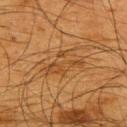follow-up: total-body-photography surveillance lesion; no biopsy
site: the upper back
imaging modality: ~15 mm tile from a whole-body skin photo
tile lighting: cross-polarized illumination
subject: male, roughly 65 years of age
automated lesion analysis: an area of roughly 9.5 mm², an eccentricity of roughly 0.85, and a symmetry-axis asymmetry near 0.45; an average lesion color of about L≈39 a*≈19 b*≈35 (CIELAB), roughly 6 lightness units darker than nearby skin, and a normalized lesion–skin contrast near 5